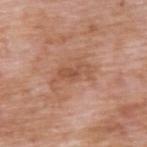Clinical summary: From the upper back. The subject is a male aged 58 to 62. A roughly 15 mm field-of-view crop from a total-body skin photograph. The recorded lesion diameter is about 5 mm.